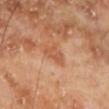Findings:
– follow-up: imaged on a skin check; not biopsied
– imaging modality: ~15 mm tile from a whole-body skin photo
– subject: male, aged approximately 70
– location: the left lower leg